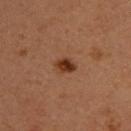Impression: Part of a total-body skin-imaging series; this lesion was reviewed on a skin check and was not flagged for biopsy. Image and clinical context: From the upper back. A male patient, aged approximately 35. Captured under cross-polarized illumination. Cropped from a total-body skin-imaging series; the visible field is about 15 mm.